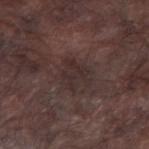Findings:
* notes: total-body-photography surveillance lesion; no biopsy
* lighting: white-light illumination
* lesion size: ~4 mm (longest diameter)
* body site: the right forearm
* image: ~15 mm crop, total-body skin-cancer survey
* subject: male, in their mid- to late 70s